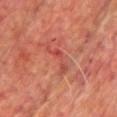This lesion was catalogued during total-body skin photography and was not selected for biopsy. A close-up tile cropped from a whole-body skin photograph, about 15 mm across. From the chest. This is a cross-polarized tile. A male subject, approximately 75 years of age.